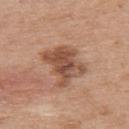– site — the upper back
– diameter — ~5 mm (longest diameter)
– subject — male, aged around 70
– tile lighting — white-light
– automated metrics — an area of roughly 16 mm², an outline eccentricity of about 0.6 (0 = round, 1 = elongated), and a shape-asymmetry score of about 0.3 (0 = symmetric); a mean CIELAB color near L≈51 a*≈21 b*≈30, about 12 CIELAB-L* units darker than the surrounding skin, and a normalized border contrast of about 8.5
– acquisition — ~15 mm crop, total-body skin-cancer survey